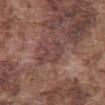This lesion was catalogued during total-body skin photography and was not selected for biopsy. Measured at roughly 3.5 mm in maximum diameter. A male patient, aged 73–77. This image is a 15 mm lesion crop taken from a total-body photograph. An algorithmic analysis of the crop reported a footprint of about 6.5 mm², an outline eccentricity of about 0.7 (0 = round, 1 = elongated), and a symmetry-axis asymmetry near 0.6. It also reported a lesion color around L≈42 a*≈20 b*≈20 in CIELAB, about 6 CIELAB-L* units darker than the surrounding skin, and a normalized border contrast of about 5.5. On the abdomen.